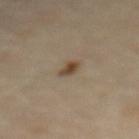This lesion was catalogued during total-body skin photography and was not selected for biopsy. A female patient in their mid- to late 60s. The tile uses cross-polarized illumination. About 2.5 mm across. A 15 mm crop from a total-body photograph taken for skin-cancer surveillance. Located on the mid back.